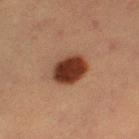Captured during whole-body skin photography for melanoma surveillance; the lesion was not biopsied. Located on the left thigh. The tile uses cross-polarized illumination. A lesion tile, about 15 mm wide, cut from a 3D total-body photograph. Approximately 4.5 mm at its widest. Automated image analysis of the tile measured an average lesion color of about L≈28 a*≈20 b*≈25 (CIELAB), roughly 16 lightness units darker than nearby skin, and a normalized lesion–skin contrast near 14.5. The software also gave a classifier nevus-likeness of about 100/100. A female patient aged approximately 55.